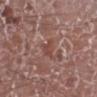{"biopsy_status": "not biopsied; imaged during a skin examination", "lighting": "white-light", "image": {"source": "total-body photography crop", "field_of_view_mm": 15}, "lesion_size": {"long_diameter_mm_approx": 2.5}, "automated_metrics": {"vs_skin_darker_L": 7.0, "vs_skin_contrast_norm": 6.0}, "patient": {"sex": "male", "age_approx": 75}, "site": "left lower leg"}